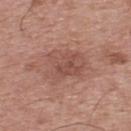| field | value |
|---|---|
| follow-up | imaged on a skin check; not biopsied |
| lighting | white-light |
| anatomic site | the upper back |
| patient | male, aged 63 to 67 |
| acquisition | total-body-photography crop, ~15 mm field of view |
| lesion size | ~5.5 mm (longest diameter) |